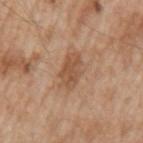  biopsy_status: not biopsied; imaged during a skin examination
  automated_metrics:
    area_mm2_approx: 8.0
    eccentricity: 0.85
    shape_asymmetry: 0.25
    cielab_L: 52
    cielab_a: 20
    cielab_b: 32
    nevus_likeness_0_100: 35
    lesion_detection_confidence_0_100: 100
  site: arm
  image:
    source: total-body photography crop
    field_of_view_mm: 15
  lighting: white-light
  patient:
    sex: male
    age_approx: 65